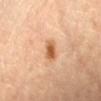Q: Is there a histopathology result?
A: catalogued during a skin exam; not biopsied
Q: Lesion size?
A: ~2.5 mm (longest diameter)
Q: Who is the patient?
A: female, in their mid- to late 60s
Q: Lesion location?
A: the right forearm
Q: What is the imaging modality?
A: total-body-photography crop, ~15 mm field of view